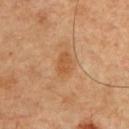notes — imaged on a skin check; not biopsied
tile lighting — cross-polarized
body site — the chest
lesion size — ~3.5 mm (longest diameter)
subject — male, aged approximately 50
image source — total-body-photography crop, ~15 mm field of view
automated metrics — an average lesion color of about L≈54 a*≈23 b*≈39 (CIELAB), a lesion–skin lightness drop of about 7, and a normalized lesion–skin contrast near 6; a nevus-likeness score of about 5/100